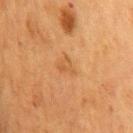Q: Is there a histopathology result?
A: imaged on a skin check; not biopsied
Q: Who is the patient?
A: female, aged approximately 55
Q: What lighting was used for the tile?
A: cross-polarized illumination
Q: How large is the lesion?
A: about 2.5 mm
Q: Lesion location?
A: the chest
Q: What kind of image is this?
A: ~15 mm crop, total-body skin-cancer survey
Q: Automated lesion metrics?
A: a color-variation rating of about 2.5/10 and peripheral color asymmetry of about 1; a classifier nevus-likeness of about 0/100 and lesion-presence confidence of about 100/100